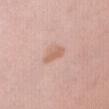The lesion was photographed on a routine skin check and not biopsied; there is no pathology result. A lesion tile, about 15 mm wide, cut from a 3D total-body photograph. The lesion is located on the front of the torso. Longest diameter approximately 3 mm. A female patient aged approximately 45.